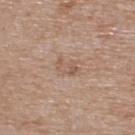Impression:
Imaged during a routine full-body skin examination; the lesion was not biopsied and no histopathology is available.
Clinical summary:
The recorded lesion diameter is about 3 mm. Cropped from a whole-body photographic skin survey; the tile spans about 15 mm. A male patient in their mid-70s. The lesion is on the upper back. Captured under white-light illumination.